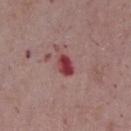{
  "biopsy_status": "not biopsied; imaged during a skin examination",
  "lighting": "white-light",
  "lesion_size": {
    "long_diameter_mm_approx": 3.0
  },
  "image": {
    "source": "total-body photography crop",
    "field_of_view_mm": 15
  },
  "patient": {
    "sex": "male",
    "age_approx": 70
  },
  "automated_metrics": {
    "area_mm2_approx": 4.5,
    "eccentricity": 0.75,
    "shape_asymmetry": 0.25,
    "vs_skin_darker_L": 14.0,
    "vs_skin_contrast_norm": 11.0
  },
  "site": "chest"
}